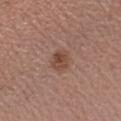No biopsy was performed on this lesion — it was imaged during a full skin examination and was not determined to be concerning. The patient is a female about 50 years old. This is a white-light tile. Automated image analysis of the tile measured border irregularity of about 2 on a 0–10 scale, a color-variation rating of about 4/10, and a peripheral color-asymmetry measure near 1.5. The lesion is located on the left forearm. Cropped from a total-body skin-imaging series; the visible field is about 15 mm.